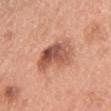No biopsy was performed on this lesion — it was imaged during a full skin examination and was not determined to be concerning.
Captured under white-light illumination.
A 15 mm close-up tile from a total-body photography series done for melanoma screening.
The recorded lesion diameter is about 5 mm.
The subject is a male about 30 years old.
The lesion is on the chest.
The lesion-visualizer software estimated a lesion area of about 14 mm², an outline eccentricity of about 0.75 (0 = round, 1 = elongated), and two-axis asymmetry of about 0.25. It also reported a nevus-likeness score of about 15/100 and a lesion-detection confidence of about 100/100.